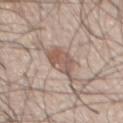Assessment: The lesion was tiled from a total-body skin photograph and was not biopsied. Acquisition and patient details: A roughly 15 mm field-of-view crop from a total-body skin photograph. A male subject, aged around 50. The lesion is on the chest.